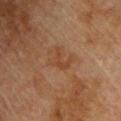Assessment: Captured during whole-body skin photography for melanoma surveillance; the lesion was not biopsied. Clinical summary: The subject is a male aged around 75. The lesion is on the upper back. A 15 mm close-up tile from a total-body photography series done for melanoma screening.